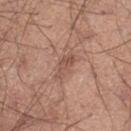The lesion was photographed on a routine skin check and not biopsied; there is no pathology result. The lesion's longest dimension is about 3.5 mm. The tile uses white-light illumination. A male subject, approximately 40 years of age. An algorithmic analysis of the crop reported a shape-asymmetry score of about 0.45 (0 = symmetric). It also reported a classifier nevus-likeness of about 0/100 and a lesion-detection confidence of about 100/100. On the left thigh. A 15 mm close-up extracted from a 3D total-body photography capture.